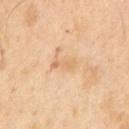image source: ~15 mm crop, total-body skin-cancer survey | patient: male, approximately 65 years of age | lesion size: ~3.5 mm (longest diameter) | illumination: cross-polarized | automated metrics: a lesion color around L≈68 a*≈18 b*≈37 in CIELAB and roughly 7 lightness units darker than nearby skin.